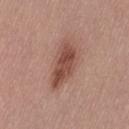Captured during whole-body skin photography for melanoma surveillance; the lesion was not biopsied.
Cropped from a whole-body photographic skin survey; the tile spans about 15 mm.
On the lower back.
Approximately 5.5 mm at its widest.
This is a white-light tile.
Automated image analysis of the tile measured an area of roughly 12 mm² and a symmetry-axis asymmetry near 0.3. The analysis additionally found a border-irregularity index near 3.5/10, a within-lesion color-variation index near 4/10, and peripheral color asymmetry of about 1.5.
A female patient roughly 50 years of age.